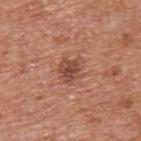Q: Was this lesion biopsied?
A: catalogued during a skin exam; not biopsied
Q: Patient demographics?
A: male, aged approximately 65
Q: What is the imaging modality?
A: total-body-photography crop, ~15 mm field of view
Q: What is the lesion's diameter?
A: about 3 mm
Q: Illumination type?
A: white-light
Q: What is the anatomic site?
A: the upper back